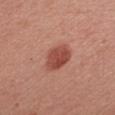| field | value |
|---|---|
| follow-up | imaged on a skin check; not biopsied |
| imaging modality | ~15 mm crop, total-body skin-cancer survey |
| automated metrics | an area of roughly 9 mm², an outline eccentricity of about 0.8 (0 = round, 1 = elongated), and two-axis asymmetry of about 0.2; a mean CIELAB color near L≈48 a*≈29 b*≈28; border irregularity of about 2 on a 0–10 scale, a color-variation rating of about 3.5/10, and radial color variation of about 1 |
| size | ≈4.5 mm |
| patient | female, approximately 40 years of age |
| location | the right upper arm |